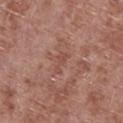Assessment: The lesion was tiled from a total-body skin photograph and was not biopsied. Clinical summary: Cropped from a total-body skin-imaging series; the visible field is about 15 mm. The lesion is on the leg. A male subject aged approximately 55.